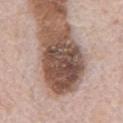Captured during whole-body skin photography for melanoma surveillance; the lesion was not biopsied.
The tile uses white-light illumination.
A male subject aged around 70.
Longest diameter approximately 8.5 mm.
Cropped from a whole-body photographic skin survey; the tile spans about 15 mm.
On the front of the torso.
The lesion-visualizer software estimated an automated nevus-likeness rating near 5 out of 100 and a lesion-detection confidence of about 85/100.